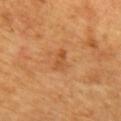Q: Is there a histopathology result?
A: catalogued during a skin exam; not biopsied
Q: How was this image acquired?
A: ~15 mm tile from a whole-body skin photo
Q: How was the tile lit?
A: cross-polarized
Q: Where on the body is the lesion?
A: the upper back
Q: What are the patient's age and sex?
A: male, aged around 65
Q: How large is the lesion?
A: ~2.5 mm (longest diameter)